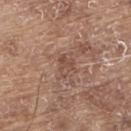Q: Was this lesion biopsied?
A: imaged on a skin check; not biopsied
Q: What is the imaging modality?
A: ~15 mm crop, total-body skin-cancer survey
Q: Illumination type?
A: white-light
Q: Automated lesion metrics?
A: a lesion area of about 3 mm², an eccentricity of roughly 0.7, and a shape-asymmetry score of about 0.45 (0 = symmetric); border irregularity of about 4.5 on a 0–10 scale, internal color variation of about 1.5 on a 0–10 scale, and a peripheral color-asymmetry measure near 0.5
Q: What are the patient's age and sex?
A: male, roughly 80 years of age
Q: What is the anatomic site?
A: the back
Q: What is the lesion's diameter?
A: ≈2.5 mm